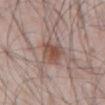{
  "biopsy_status": "not biopsied; imaged during a skin examination",
  "patient": {
    "sex": "male",
    "age_approx": 60
  },
  "site": "abdomen",
  "image": {
    "source": "total-body photography crop",
    "field_of_view_mm": 15
  }
}